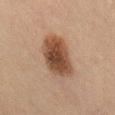The lesion was photographed on a routine skin check and not biopsied; there is no pathology result. Automated image analysis of the tile measured an outline eccentricity of about 0.75 (0 = round, 1 = elongated) and two-axis asymmetry of about 0.15. And it measured a lesion color around L≈38 a*≈17 b*≈26 in CIELAB, a lesion–skin lightness drop of about 13, and a normalized lesion–skin contrast near 11. The analysis additionally found internal color variation of about 4.5 on a 0–10 scale and radial color variation of about 1. From the lower back. A 15 mm close-up extracted from a 3D total-body photography capture. The patient is a female aged 38 to 42.